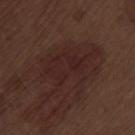Q: Is there a histopathology result?
A: catalogued during a skin exam; not biopsied
Q: How was this image acquired?
A: ~15 mm tile from a whole-body skin photo
Q: What is the anatomic site?
A: the left thigh
Q: Patient demographics?
A: male, aged around 70
Q: What did automated image analysis measure?
A: a lesion–skin lightness drop of about 5 and a normalized border contrast of about 6; a classifier nevus-likeness of about 5/100
Q: What is the lesion's diameter?
A: about 8.5 mm